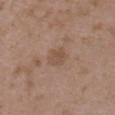lighting — white-light
patient — female, about 35 years old
lesion diameter — ≈2.5 mm
image — ~15 mm crop, total-body skin-cancer survey
body site — the upper back